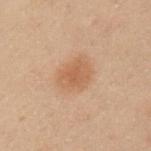- notes — total-body-photography surveillance lesion; no biopsy
- lighting — cross-polarized
- patient — male, roughly 55 years of age
- diameter — ≈4 mm
- imaging modality — total-body-photography crop, ~15 mm field of view
- location — the arm
- TBP lesion metrics — a lesion color around L≈48 a*≈16 b*≈29 in CIELAB; internal color variation of about 2 on a 0–10 scale and a peripheral color-asymmetry measure near 0.5; a nevus-likeness score of about 95/100 and a lesion-detection confidence of about 100/100